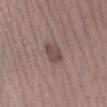Recorded during total-body skin imaging; not selected for excision or biopsy. A female patient, aged around 50. The total-body-photography lesion software estimated a lesion area of about 5 mm², an eccentricity of roughly 0.8, and two-axis asymmetry of about 0.2. And it measured a lesion color around L≈46 a*≈17 b*≈20 in CIELAB, roughly 9 lightness units darker than nearby skin, and a normalized lesion–skin contrast near 7. The analysis additionally found a color-variation rating of about 2/10 and peripheral color asymmetry of about 0.5. And it measured lesion-presence confidence of about 100/100. On the left lower leg. A region of skin cropped from a whole-body photographic capture, roughly 15 mm wide. Approximately 3 mm at its widest. Imaged with white-light lighting.